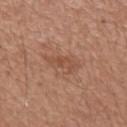Context:
Approximately 3.5 mm at its widest. A male subject, aged around 65. The total-body-photography lesion software estimated a mean CIELAB color near L≈50 a*≈22 b*≈31, about 7 CIELAB-L* units darker than the surrounding skin, and a normalized border contrast of about 5.5. It also reported a border-irregularity rating of about 6.5/10 and radial color variation of about 0. A 15 mm close-up tile from a total-body photography series done for melanoma screening. Captured under white-light illumination. On the back.